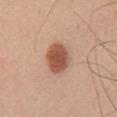– biopsy status: no biopsy performed (imaged during a skin exam)